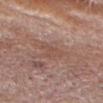notes: no biopsy performed (imaged during a skin exam) | automated lesion analysis: an average lesion color of about L≈51 a*≈19 b*≈26 (CIELAB) and a lesion-to-skin contrast of about 5 (normalized; higher = more distinct); an automated nevus-likeness rating near 0 out of 100 and a lesion-detection confidence of about 70/100 | body site: the head or neck | image: total-body-photography crop, ~15 mm field of view | patient: male, aged approximately 65 | lighting: white-light | size: ≈4 mm.